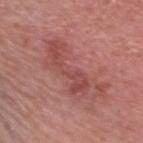Q: Is there a histopathology result?
A: no biopsy performed (imaged during a skin exam)
Q: Lesion size?
A: ~8.5 mm (longest diameter)
Q: What is the imaging modality?
A: 15 mm crop, total-body photography
Q: What is the anatomic site?
A: the head or neck
Q: Patient demographics?
A: male, aged approximately 60